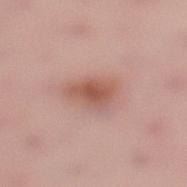This lesion was catalogued during total-body skin photography and was not selected for biopsy.
Cropped from a total-body skin-imaging series; the visible field is about 15 mm.
A female subject aged 23–27.
Imaged with white-light lighting.
About 4.5 mm across.
Located on the left lower leg.